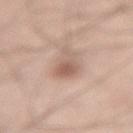Clinical summary: A male patient aged approximately 60. Located on the lower back. Automated image analysis of the tile measured an average lesion color of about L≈59 a*≈19 b*≈26 (CIELAB), about 11 CIELAB-L* units darker than the surrounding skin, and a normalized lesion–skin contrast near 7. The software also gave an automated nevus-likeness rating near 35 out of 100 and a detector confidence of about 100 out of 100 that the crop contains a lesion. A 15 mm close-up tile from a total-body photography series done for melanoma screening.